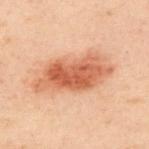This lesion was catalogued during total-body skin photography and was not selected for biopsy. A roughly 15 mm field-of-view crop from a total-body skin photograph. The lesion-visualizer software estimated a border-irregularity rating of about 3.5/10, internal color variation of about 5.5 on a 0–10 scale, and a peripheral color-asymmetry measure near 1.5. The analysis additionally found an automated nevus-likeness rating near 95 out of 100. The lesion is located on the upper back. The recorded lesion diameter is about 8.5 mm. The subject is a male roughly 50 years of age. The tile uses cross-polarized illumination.